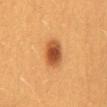A female patient, aged around 30.
On the abdomen.
A 15 mm crop from a total-body photograph taken for skin-cancer surveillance.